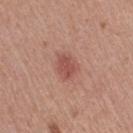notes: no biopsy performed (imaged during a skin exam) | imaging modality: ~15 mm tile from a whole-body skin photo | body site: the right thigh | diameter: ≈3.5 mm | subject: female, approximately 55 years of age | illumination: white-light illumination.